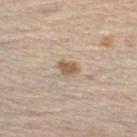Part of a total-body skin-imaging series; this lesion was reviewed on a skin check and was not flagged for biopsy. A 15 mm close-up extracted from a 3D total-body photography capture. On the leg. Imaged with white-light lighting. Measured at roughly 2.5 mm in maximum diameter. A male subject in their 70s.